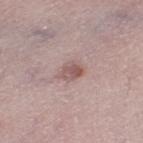Assessment:
Recorded during total-body skin imaging; not selected for excision or biopsy.
Acquisition and patient details:
The tile uses white-light illumination. A roughly 15 mm field-of-view crop from a total-body skin photograph. From the left thigh. Longest diameter approximately 2.5 mm. A female patient, aged around 40.